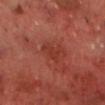Q: Was this lesion biopsied?
A: no biopsy performed (imaged during a skin exam)
Q: How large is the lesion?
A: about 3 mm
Q: What kind of image is this?
A: ~15 mm crop, total-body skin-cancer survey
Q: What are the patient's age and sex?
A: male, aged approximately 70
Q: What lighting was used for the tile?
A: cross-polarized illumination
Q: What did automated image analysis measure?
A: a mean CIELAB color near L≈36 a*≈30 b*≈30, a lesion–skin lightness drop of about 5, and a normalized border contrast of about 5.5; border irregularity of about 4 on a 0–10 scale, a within-lesion color-variation index near 1/10, and a peripheral color-asymmetry measure near 0.5
Q: Where on the body is the lesion?
A: the chest